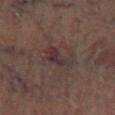notes=total-body-photography surveillance lesion; no biopsy
image=~15 mm crop, total-body skin-cancer survey
automated metrics=an area of roughly 7 mm² and two-axis asymmetry of about 0.4
lighting=cross-polarized illumination
subject=male, aged approximately 70
lesion size=~3.5 mm (longest diameter)
location=the left lower leg